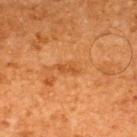follow-up — imaged on a skin check; not biopsied
anatomic site — the upper back
image — 15 mm crop, total-body photography
patient — male, aged 63 to 67